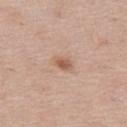Clinical impression: Part of a total-body skin-imaging series; this lesion was reviewed on a skin check and was not flagged for biopsy. Image and clinical context: Longest diameter approximately 2.5 mm. Imaged with white-light lighting. On the left thigh. This image is a 15 mm lesion crop taken from a total-body photograph. A female subject, aged around 40. The lesion-visualizer software estimated a mean CIELAB color near L≈58 a*≈21 b*≈30, a lesion–skin lightness drop of about 10, and a lesion-to-skin contrast of about 7 (normalized; higher = more distinct). And it measured a border-irregularity index near 2.5/10 and a peripheral color-asymmetry measure near 0.5. And it measured a nevus-likeness score of about 60/100 and a lesion-detection confidence of about 100/100.